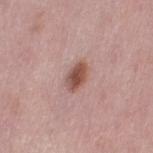{
  "biopsy_status": "not biopsied; imaged during a skin examination",
  "lighting": "white-light",
  "lesion_size": {
    "long_diameter_mm_approx": 3.0
  },
  "image": {
    "source": "total-body photography crop",
    "field_of_view_mm": 15
  },
  "site": "left thigh",
  "patient": {
    "sex": "female",
    "age_approx": 50
  },
  "automated_metrics": {
    "nevus_likeness_0_100": 95,
    "lesion_detection_confidence_0_100": 100
  }
}